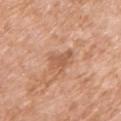<record>
<biopsy_status>not biopsied; imaged during a skin examination</biopsy_status>
<patient>
  <sex>female</sex>
  <age_approx>75</age_approx>
</patient>
<lighting>white-light</lighting>
<lesion_size>
  <long_diameter_mm_approx>3.5</long_diameter_mm_approx>
</lesion_size>
<site>right upper arm</site>
<image>
  <source>total-body photography crop</source>
  <field_of_view_mm>15</field_of_view_mm>
</image>
</record>